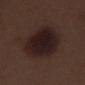Q: Was this lesion biopsied?
A: total-body-photography surveillance lesion; no biopsy
Q: How was the tile lit?
A: white-light
Q: What is the anatomic site?
A: the right thigh
Q: Who is the patient?
A: male, aged around 70
Q: What is the imaging modality?
A: total-body-photography crop, ~15 mm field of view
Q: What is the lesion's diameter?
A: ≈6.5 mm